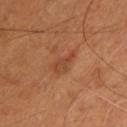Q: Was this lesion biopsied?
A: imaged on a skin check; not biopsied
Q: Illumination type?
A: cross-polarized illumination
Q: What did automated image analysis measure?
A: an outline eccentricity of about 0.85 (0 = round, 1 = elongated) and two-axis asymmetry of about 0.45; a border-irregularity rating of about 4.5/10 and internal color variation of about 2 on a 0–10 scale; lesion-presence confidence of about 100/100
Q: What kind of image is this?
A: total-body-photography crop, ~15 mm field of view
Q: Lesion location?
A: the chest
Q: What are the patient's age and sex?
A: male, about 55 years old
Q: How large is the lesion?
A: about 3.5 mm